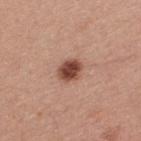biopsy_status: not biopsied; imaged during a skin examination
site: upper back
patient:
  sex: male
  age_approx: 40
image:
  source: total-body photography crop
  field_of_view_mm: 15